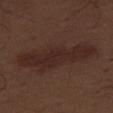The lesion was photographed on a routine skin check and not biopsied; there is no pathology result. The lesion is on the right thigh. A male patient in their 70s. Cropped from a whole-body photographic skin survey; the tile spans about 15 mm. Automated image analysis of the tile measured a footprint of about 22 mm², a shape eccentricity near 0.95, and a symmetry-axis asymmetry near 0.3. The analysis additionally found a border-irregularity index near 5.5/10, a within-lesion color-variation index near 2/10, and a peripheral color-asymmetry measure near 1. The analysis additionally found a lesion-detection confidence of about 80/100. This is a white-light tile.